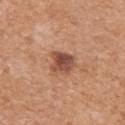follow-up: imaged on a skin check; not biopsied
TBP lesion metrics: an area of roughly 7 mm² and a shape-asymmetry score of about 0.25 (0 = symmetric); border irregularity of about 2.5 on a 0–10 scale, a within-lesion color-variation index near 5.5/10, and peripheral color asymmetry of about 2; a lesion-detection confidence of about 100/100
site: the left upper arm
subject: female, in their mid- to late 50s
illumination: white-light illumination
acquisition: ~15 mm crop, total-body skin-cancer survey